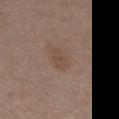No biopsy was performed on this lesion — it was imaged during a full skin examination and was not determined to be concerning.
A 15 mm close-up extracted from a 3D total-body photography capture.
From the chest.
Automated tile analysis of the lesion measured a lesion color around L≈47 a*≈16 b*≈26 in CIELAB, a lesion–skin lightness drop of about 6, and a lesion-to-skin contrast of about 5.5 (normalized; higher = more distinct). The software also gave border irregularity of about 3.5 on a 0–10 scale and peripheral color asymmetry of about 0.
This is a white-light tile.
The lesion's longest dimension is about 3 mm.
A male patient, in their mid-50s.